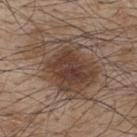follow-up — no biopsy performed (imaged during a skin exam)
lesion size — ≈8.5 mm
image — ~15 mm crop, total-body skin-cancer survey
image-analysis metrics — internal color variation of about 5 on a 0–10 scale and radial color variation of about 1.5; an automated nevus-likeness rating near 90 out of 100 and a detector confidence of about 100 out of 100 that the crop contains a lesion
location — the back
illumination — white-light illumination
subject — male, aged 43–47